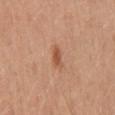A male patient roughly 30 years of age.
This is a white-light tile.
The lesion-visualizer software estimated a footprint of about 2.5 mm², an eccentricity of roughly 0.9, and a shape-asymmetry score of about 0.25 (0 = symmetric). The analysis additionally found a border-irregularity rating of about 2.5/10, internal color variation of about 0 on a 0–10 scale, and radial color variation of about 0. And it measured a lesion-detection confidence of about 100/100.
Measured at roughly 2.5 mm in maximum diameter.
From the mid back.
A roughly 15 mm field-of-view crop from a total-body skin photograph.